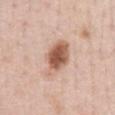This lesion was catalogued during total-body skin photography and was not selected for biopsy. Captured under white-light illumination. About 5 mm across. Automated image analysis of the tile measured a lesion area of about 12 mm², a shape eccentricity near 0.8, and two-axis asymmetry of about 0.2. The analysis additionally found a lesion color around L≈59 a*≈21 b*≈30 in CIELAB, about 15 CIELAB-L* units darker than the surrounding skin, and a normalized border contrast of about 10. It also reported a border-irregularity index near 2/10 and peripheral color asymmetry of about 1.5. The software also gave a nevus-likeness score of about 100/100 and lesion-presence confidence of about 100/100. Located on the abdomen. A roughly 15 mm field-of-view crop from a total-body skin photograph. A male subject aged around 50.